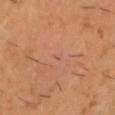follow-up: catalogued during a skin exam; not biopsied
subject: male, in their mid- to late 40s
imaging modality: ~15 mm tile from a whole-body skin photo
illumination: cross-polarized
TBP lesion metrics: an area of roughly 0.5 mm², an eccentricity of roughly 0.95, and a symmetry-axis asymmetry near 0.4; a lesion color around L≈54 a*≈24 b*≈31 in CIELAB, about 5 CIELAB-L* units darker than the surrounding skin, and a lesion-to-skin contrast of about 3.5 (normalized; higher = more distinct); a detector confidence of about 90 out of 100 that the crop contains a lesion
body site: the head or neck
lesion diameter: about 1.5 mm